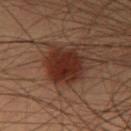Impression:
Recorded during total-body skin imaging; not selected for excision or biopsy.
Clinical summary:
Cropped from a total-body skin-imaging series; the visible field is about 15 mm. The lesion is located on the chest. Imaged with cross-polarized lighting. The subject is a male aged 53–57.